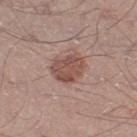follow-up: catalogued during a skin exam; not biopsied
anatomic site: the left thigh
size: ≈4 mm
lighting: white-light
subject: male, in their 50s
image-analysis metrics: a footprint of about 10 mm², an outline eccentricity of about 0.6 (0 = round, 1 = elongated), and two-axis asymmetry of about 0.2; about 10 CIELAB-L* units darker than the surrounding skin; an automated nevus-likeness rating near 70 out of 100 and a lesion-detection confidence of about 100/100
acquisition: ~15 mm crop, total-body skin-cancer survey